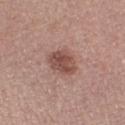follow-up=catalogued during a skin exam; not biopsied | subject=female, aged 48 to 52 | TBP lesion metrics=an area of roughly 8.5 mm² and two-axis asymmetry of about 0.2; a classifier nevus-likeness of about 70/100 | image=total-body-photography crop, ~15 mm field of view | location=the left forearm | tile lighting=white-light illumination | lesion size=~3.5 mm (longest diameter).